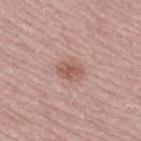Clinical impression:
Recorded during total-body skin imaging; not selected for excision or biopsy.
Acquisition and patient details:
Captured under white-light illumination. A lesion tile, about 15 mm wide, cut from a 3D total-body photograph. The lesion-visualizer software estimated an area of roughly 6.5 mm² and a shape-asymmetry score of about 0.2 (0 = symmetric). It also reported a mean CIELAB color near L≈57 a*≈21 b*≈26. And it measured border irregularity of about 1.5 on a 0–10 scale, a color-variation rating of about 3/10, and peripheral color asymmetry of about 1. The software also gave a nevus-likeness score of about 65/100 and lesion-presence confidence of about 100/100. On the right thigh. A female subject, in their 60s. The recorded lesion diameter is about 3.5 mm.